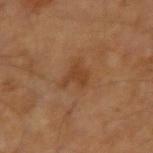biopsy status: catalogued during a skin exam; not biopsied | lesion size: ~3 mm (longest diameter) | subject: male, aged approximately 65 | site: the arm | acquisition: ~15 mm tile from a whole-body skin photo | lighting: cross-polarized.